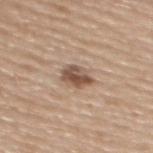Assessment:
Captured during whole-body skin photography for melanoma surveillance; the lesion was not biopsied.
Clinical summary:
The subject is a female aged around 70. A roughly 15 mm field-of-view crop from a total-body skin photograph. This is a white-light tile. The lesion is on the upper back. The lesion's longest dimension is about 3.5 mm.